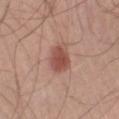Captured during whole-body skin photography for melanoma surveillance; the lesion was not biopsied.
On the right lower leg.
A male patient about 50 years old.
This image is a 15 mm lesion crop taken from a total-body photograph.